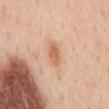{"biopsy_status": "not biopsied; imaged during a skin examination", "lesion_size": {"long_diameter_mm_approx": 3.5}, "site": "back", "lighting": "white-light", "patient": {"sex": "female", "age_approx": 45}, "image": {"source": "total-body photography crop", "field_of_view_mm": 15}}